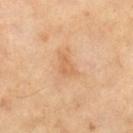| key | value |
|---|---|
| workup | catalogued during a skin exam; not biopsied |
| image | ~15 mm crop, total-body skin-cancer survey |
| subject | male, in their mid- to late 60s |